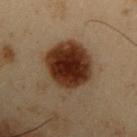Impression:
Part of a total-body skin-imaging series; this lesion was reviewed on a skin check and was not flagged for biopsy.
Clinical summary:
This image is a 15 mm lesion crop taken from a total-body photograph. This is a cross-polarized tile. A male subject, approximately 55 years of age. The total-body-photography lesion software estimated a lesion area of about 29 mm² and a symmetry-axis asymmetry near 0.2. It also reported a border-irregularity rating of about 2.5/10, internal color variation of about 7.5 on a 0–10 scale, and radial color variation of about 2. The software also gave a classifier nevus-likeness of about 100/100. The lesion is located on the left upper arm.